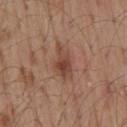{"biopsy_status": "not biopsied; imaged during a skin examination", "site": "mid back", "lesion_size": {"long_diameter_mm_approx": 4.5}, "patient": {"sex": "male", "age_approx": 55}, "lighting": "white-light", "image": {"source": "total-body photography crop", "field_of_view_mm": 15}}